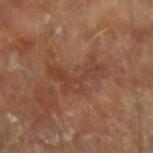Findings:
• workup · no biopsy performed (imaged during a skin exam)
• anatomic site · the left forearm
• image source · ~15 mm tile from a whole-body skin photo
• subject · male, about 65 years old
• size · about 6 mm
• illumination · cross-polarized illumination
• automated lesion analysis · a footprint of about 9.5 mm², an eccentricity of roughly 0.85, and a symmetry-axis asymmetry near 0.8; a border-irregularity rating of about 10/10, a within-lesion color-variation index near 4.5/10, and a peripheral color-asymmetry measure near 1.5; an automated nevus-likeness rating near 0 out of 100 and a lesion-detection confidence of about 95/100